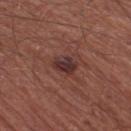Captured during whole-body skin photography for melanoma surveillance; the lesion was not biopsied. Automated tile analysis of the lesion measured a lesion area of about 5 mm², an outline eccentricity of about 0.6 (0 = round, 1 = elongated), and two-axis asymmetry of about 0.3. And it measured a detector confidence of about 100 out of 100 that the crop contains a lesion. Approximately 3 mm at its widest. This is a white-light tile. From the right thigh. A 15 mm crop from a total-body photograph taken for skin-cancer surveillance. The subject is a male aged approximately 65.